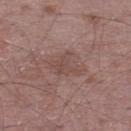notes: imaged on a skin check; not biopsied.